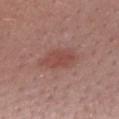The lesion was photographed on a routine skin check and not biopsied; there is no pathology result. Automated image analysis of the tile measured an eccentricity of roughly 0.85 and a shape-asymmetry score of about 0.25 (0 = symmetric). The analysis additionally found an automated nevus-likeness rating near 70 out of 100 and a detector confidence of about 100 out of 100 that the crop contains a lesion. A close-up tile cropped from a whole-body skin photograph, about 15 mm across. The patient is a female aged 23–27. On the chest. The recorded lesion diameter is about 5 mm.